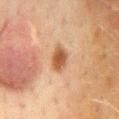follow-up: no biopsy performed (imaged during a skin exam); lesion diameter: ~3 mm (longest diameter); body site: the mid back; tile lighting: cross-polarized illumination; imaging modality: ~15 mm tile from a whole-body skin photo; subject: female, approximately 50 years of age.